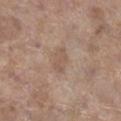The lesion was tiled from a total-body skin photograph and was not biopsied. From the leg. A 15 mm close-up tile from a total-body photography series done for melanoma screening. A female subject, aged approximately 85 or older.